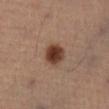Impression: Recorded during total-body skin imaging; not selected for excision or biopsy. Context: A male subject, aged 48 to 52. Located on the left leg. A roughly 15 mm field-of-view crop from a total-body skin photograph.